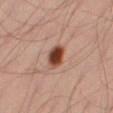Impression: Imaged during a routine full-body skin examination; the lesion was not biopsied and no histopathology is available. Acquisition and patient details: A male patient roughly 35 years of age. From the right thigh. A region of skin cropped from a whole-body photographic capture, roughly 15 mm wide.